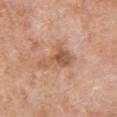Acquisition and patient details:
Imaged with white-light lighting. The lesion is on the front of the torso. A 15 mm crop from a total-body photograph taken for skin-cancer surveillance. A female subject, roughly 60 years of age. The lesion's longest dimension is about 5 mm.